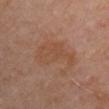Captured during whole-body skin photography for melanoma surveillance; the lesion was not biopsied. On the left upper arm. A female patient, about 50 years old. A region of skin cropped from a whole-body photographic capture, roughly 15 mm wide.